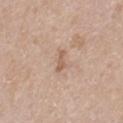Impression:
Part of a total-body skin-imaging series; this lesion was reviewed on a skin check and was not flagged for biopsy.
Acquisition and patient details:
The total-body-photography lesion software estimated a lesion area of about 3 mm², an eccentricity of roughly 0.9, and a shape-asymmetry score of about 0.4 (0 = symmetric). And it measured a classifier nevus-likeness of about 0/100. A 15 mm crop from a total-body photograph taken for skin-cancer surveillance. From the upper back. Imaged with white-light lighting. A male patient, aged 38–42.